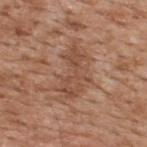workup=total-body-photography surveillance lesion; no biopsy
illumination=white-light illumination
patient=male, aged around 65
anatomic site=the upper back
image=15 mm crop, total-body photography